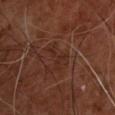Captured during whole-body skin photography for melanoma surveillance; the lesion was not biopsied.
The patient is a male in their mid-50s.
A 15 mm close-up tile from a total-body photography series done for melanoma screening.
Located on the back.